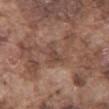workup — catalogued during a skin exam; not biopsied
lesion size — ~3 mm (longest diameter)
lighting — white-light
body site — the chest
image — ~15 mm crop, total-body skin-cancer survey
subject — male, aged 73 to 77